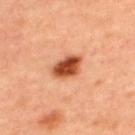follow-up: no biopsy performed (imaged during a skin exam) | tile lighting: cross-polarized illumination | subject: female, aged 43 to 47 | anatomic site: the back | acquisition: total-body-photography crop, ~15 mm field of view.